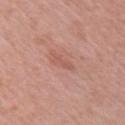* notes: total-body-photography surveillance lesion; no biopsy
* subject: female, aged approximately 60
* acquisition: ~15 mm tile from a whole-body skin photo
* lesion diameter: ~3 mm (longest diameter)
* image-analysis metrics: two-axis asymmetry of about 0.45; a border-irregularity rating of about 4.5/10 and peripheral color asymmetry of about 0; an automated nevus-likeness rating near 0 out of 100 and a lesion-detection confidence of about 100/100
* site: the arm